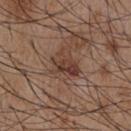| field | value |
|---|---|
| notes | no biopsy performed (imaged during a skin exam) |
| anatomic site | the chest |
| image source | total-body-photography crop, ~15 mm field of view |
| subject | male, roughly 55 years of age |